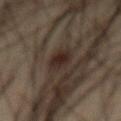  biopsy_status: not biopsied; imaged during a skin examination
  automated_metrics:
    area_mm2_approx: 6.5
    shape_asymmetry: 0.15
    vs_skin_darker_L: 8.0
    vs_skin_contrast_norm: 9.0
    nevus_likeness_0_100: 100
    lesion_detection_confidence_0_100: 100
  lesion_size:
    long_diameter_mm_approx: 4.0
  lighting: cross-polarized
  site: abdomen
  image:
    source: total-body photography crop
    field_of_view_mm: 15
  patient:
    sex: male
    age_approx: 35